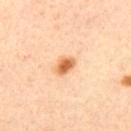Imaged during a routine full-body skin examination; the lesion was not biopsied and no histopathology is available.
The lesion is located on the back.
The tile uses cross-polarized illumination.
Automated image analysis of the tile measured an outline eccentricity of about 0.75 (0 = round, 1 = elongated) and a symmetry-axis asymmetry near 0.25. It also reported a mean CIELAB color near L≈65 a*≈24 b*≈41 and a lesion–skin lightness drop of about 14. The analysis additionally found a classifier nevus-likeness of about 100/100 and lesion-presence confidence of about 100/100.
A male patient, in their mid- to late 30s.
A lesion tile, about 15 mm wide, cut from a 3D total-body photograph.
Approximately 3 mm at its widest.